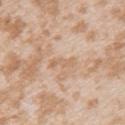Q: Was a biopsy performed?
A: imaged on a skin check; not biopsied
Q: Illumination type?
A: white-light illumination
Q: Lesion location?
A: the arm
Q: Patient demographics?
A: female, roughly 25 years of age
Q: What is the lesion's diameter?
A: ≈3 mm
Q: What is the imaging modality?
A: total-body-photography crop, ~15 mm field of view